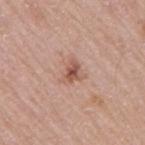<case>
  <lighting>white-light</lighting>
  <site>right upper arm</site>
  <automated_metrics>
    <cielab_L>55</cielab_L>
    <cielab_a>21</cielab_a>
    <cielab_b>28</cielab_b>
    <vs_skin_contrast_norm>7.0</vs_skin_contrast_norm>
    <border_irregularity_0_10>4.0</border_irregularity_0_10>
    <color_variation_0_10>5.0</color_variation_0_10>
  </automated_metrics>
  <image>
    <source>total-body photography crop</source>
    <field_of_view_mm>15</field_of_view_mm>
  </image>
  <lesion_size>
    <long_diameter_mm_approx>3.0</long_diameter_mm_approx>
  </lesion_size>
  <patient>
    <sex>male</sex>
    <age_approx>75</age_approx>
  </patient>
</case>